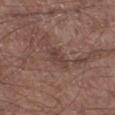Impression:
The lesion was tiled from a total-body skin photograph and was not biopsied.
Acquisition and patient details:
The recorded lesion diameter is about 3 mm. This is a white-light tile. A male subject in their mid-50s. From the right lower leg. This image is a 15 mm lesion crop taken from a total-body photograph.